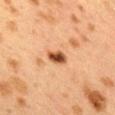Clinical impression:
This lesion was catalogued during total-body skin photography and was not selected for biopsy.
Acquisition and patient details:
A female patient aged around 40. This is a cross-polarized tile. Automated image analysis of the tile measured an area of roughly 4 mm², an outline eccentricity of about 0.8 (0 = round, 1 = elongated), and two-axis asymmetry of about 0.25. The software also gave an average lesion color of about L≈43 a*≈22 b*≈33 (CIELAB), a lesion–skin lightness drop of about 16, and a normalized border contrast of about 12. Approximately 2.5 mm at its widest. The lesion is located on the upper back. A close-up tile cropped from a whole-body skin photograph, about 15 mm across.